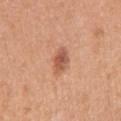<tbp_lesion>
  <biopsy_status>not biopsied; imaged during a skin examination</biopsy_status>
  <image>
    <source>total-body photography crop</source>
    <field_of_view_mm>15</field_of_view_mm>
  </image>
  <patient>
    <sex>female</sex>
    <age_approx>35</age_approx>
  </patient>
  <lighting>white-light</lighting>
  <lesion_size>
    <long_diameter_mm_approx>3.0</long_diameter_mm_approx>
  </lesion_size>
  <site>right upper arm</site>
</tbp_lesion>